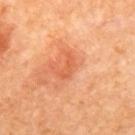Part of a total-body skin-imaging series; this lesion was reviewed on a skin check and was not flagged for biopsy. Imaged with cross-polarized lighting. Measured at roughly 3 mm in maximum diameter. The patient is a female approximately 50 years of age. From the upper back. Cropped from a total-body skin-imaging series; the visible field is about 15 mm.